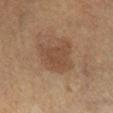No biopsy was performed on this lesion — it was imaged during a full skin examination and was not determined to be concerning.